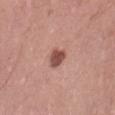Clinical impression:
The lesion was tiled from a total-body skin photograph and was not biopsied.
Image and clinical context:
A female patient aged approximately 40. Automated image analysis of the tile measured a nevus-likeness score of about 95/100. The lesion is on the lower back. This image is a 15 mm lesion crop taken from a total-body photograph. The lesion's longest dimension is about 2.5 mm. The tile uses white-light illumination.